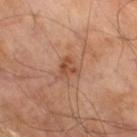Impression:
The lesion was tiled from a total-body skin photograph and was not biopsied.
Background:
From the right thigh. A lesion tile, about 15 mm wide, cut from a 3D total-body photograph. Automated tile analysis of the lesion measured a lesion color around L≈49 a*≈23 b*≈32 in CIELAB, roughly 8 lightness units darker than nearby skin, and a normalized border contrast of about 6.5. The analysis additionally found a lesion-detection confidence of about 100/100. A male subject, aged around 70. Approximately 2.5 mm at its widest.